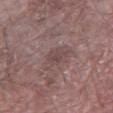biopsy status — imaged on a skin check; not biopsied
image — ~15 mm crop, total-body skin-cancer survey
patient — male, roughly 85 years of age or older
location — the right forearm
TBP lesion metrics — a lesion area of about 3.5 mm², a shape eccentricity near 0.7, and a shape-asymmetry score of about 0.25 (0 = symmetric); a lesion–skin lightness drop of about 7 and a lesion-to-skin contrast of about 5.5 (normalized; higher = more distinct); an automated nevus-likeness rating near 0 out of 100 and lesion-presence confidence of about 95/100